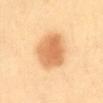* biopsy status — imaged on a skin check; not biopsied
* location — the abdomen
* image-analysis metrics — a footprint of about 16 mm², a shape eccentricity near 0.65, and a shape-asymmetry score of about 0.15 (0 = symmetric); roughly 13 lightness units darker than nearby skin
* image — 15 mm crop, total-body photography
* patient — female, aged 48 to 52
* lesion diameter — ~5 mm (longest diameter)
* tile lighting — cross-polarized illumination